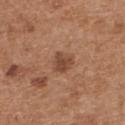Imaged during a routine full-body skin examination; the lesion was not biopsied and no histopathology is available. A 15 mm crop from a total-body photograph taken for skin-cancer surveillance. A male subject, aged approximately 70. On the back. The lesion-visualizer software estimated a lesion area of about 5 mm², a shape eccentricity near 0.35, and a symmetry-axis asymmetry near 0.2. It also reported an average lesion color of about L≈46 a*≈22 b*≈30 (CIELAB) and about 10 CIELAB-L* units darker than the surrounding skin. The software also gave border irregularity of about 2 on a 0–10 scale, a within-lesion color-variation index near 1.5/10, and a peripheral color-asymmetry measure near 0.5.